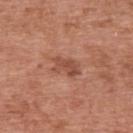Impression: No biopsy was performed on this lesion — it was imaged during a full skin examination and was not determined to be concerning. Clinical summary: Approximately 3.5 mm at its widest. A male patient, aged 68 to 72. Captured under white-light illumination. The lesion-visualizer software estimated an outline eccentricity of about 0.8 (0 = round, 1 = elongated) and a symmetry-axis asymmetry near 0.25. The analysis additionally found an average lesion color of about L≈50 a*≈25 b*≈30 (CIELAB) and about 9 CIELAB-L* units darker than the surrounding skin. It also reported a border-irregularity rating of about 3.5/10, a color-variation rating of about 2.5/10, and peripheral color asymmetry of about 1. A roughly 15 mm field-of-view crop from a total-body skin photograph. The lesion is located on the upper back.